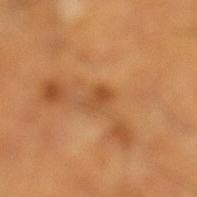| key | value |
|---|---|
| workup | total-body-photography surveillance lesion; no biopsy |
| patient | male, in their mid-50s |
| site | the left lower leg |
| automated metrics | a footprint of about 3.5 mm² and an eccentricity of roughly 0.75; a within-lesion color-variation index near 3.5/10 |
| size | about 2.5 mm |
| image source | ~15 mm crop, total-body skin-cancer survey |